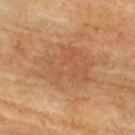Notes:
– workup — total-body-photography surveillance lesion; no biopsy
– lesion size — ~6.5 mm (longest diameter)
– anatomic site — the upper back
– patient — male, roughly 75 years of age
– image source — 15 mm crop, total-body photography
– tile lighting — cross-polarized illumination